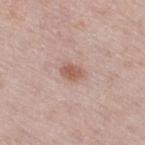The lesion was photographed on a routine skin check and not biopsied; there is no pathology result. A close-up tile cropped from a whole-body skin photograph, about 15 mm across. The tile uses white-light illumination. A female patient aged 58 to 62. Located on the right thigh. The lesion's longest dimension is about 2.5 mm. Automated tile analysis of the lesion measured about 10 CIELAB-L* units darker than the surrounding skin and a lesion-to-skin contrast of about 7.5 (normalized; higher = more distinct). And it measured border irregularity of about 2 on a 0–10 scale, a color-variation rating of about 2/10, and peripheral color asymmetry of about 0.5.